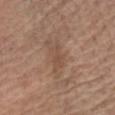Part of a total-body skin-imaging series; this lesion was reviewed on a skin check and was not flagged for biopsy.
Cropped from a whole-body photographic skin survey; the tile spans about 15 mm.
On the chest.
The patient is a male approximately 65 years of age.